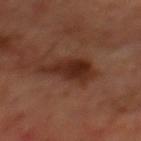Q: Was a biopsy performed?
A: catalogued during a skin exam; not biopsied
Q: What is the anatomic site?
A: the mid back
Q: What kind of image is this?
A: 15 mm crop, total-body photography
Q: Patient demographics?
A: male, in their 70s